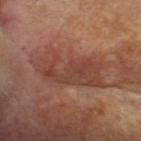Assessment: Captured during whole-body skin photography for melanoma surveillance; the lesion was not biopsied. Clinical summary: Imaged with cross-polarized lighting. A female subject aged approximately 55. Located on the head or neck. Cropped from a total-body skin-imaging series; the visible field is about 15 mm. Measured at roughly 8 mm in maximum diameter. Automated tile analysis of the lesion measured a mean CIELAB color near L≈43 a*≈23 b*≈27, about 8 CIELAB-L* units darker than the surrounding skin, and a normalized border contrast of about 6.5. It also reported a border-irregularity index near 6/10, a color-variation rating of about 4.5/10, and radial color variation of about 1.5.